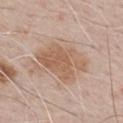biopsy_status: not biopsied; imaged during a skin examination
lesion_size:
  long_diameter_mm_approx: 6.5
lighting: white-light
automated_metrics:
  area_mm2_approx: 19.0
  shape_asymmetry: 0.3
patient:
  sex: male
  age_approx: 70
image:
  source: total-body photography crop
  field_of_view_mm: 15
site: chest